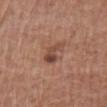Part of a total-body skin-imaging series; this lesion was reviewed on a skin check and was not flagged for biopsy. The lesion is on the chest. This image is a 15 mm lesion crop taken from a total-body photograph. A female patient aged 73 to 77.